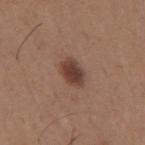Recorded during total-body skin imaging; not selected for excision or biopsy.
The subject is a male in their mid-60s.
This is a white-light tile.
The recorded lesion diameter is about 3.5 mm.
This image is a 15 mm lesion crop taken from a total-body photograph.
From the mid back.